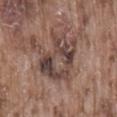  automated_metrics:
    cielab_L: 43
    cielab_a: 16
    cielab_b: 21
    vs_skin_darker_L: 10.0
    vs_skin_contrast_norm: 8.0
    border_irregularity_0_10: 5.5
    color_variation_0_10: 9.5
    peripheral_color_asymmetry: 3.5
    nevus_likeness_0_100: 5
    lesion_detection_confidence_0_100: 85
  patient:
    sex: male
    age_approx: 75
  site: back
  image:
    source: total-body photography crop
    field_of_view_mm: 15